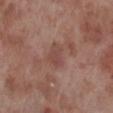Recorded during total-body skin imaging; not selected for excision or biopsy. A male patient, aged approximately 70. Automated tile analysis of the lesion measured a shape eccentricity near 0.7 and two-axis asymmetry of about 0.3. It also reported a border-irregularity rating of about 2.5/10 and a peripheral color-asymmetry measure near 1. The software also gave an automated nevus-likeness rating near 0 out of 100 and lesion-presence confidence of about 100/100. This is a white-light tile. The recorded lesion diameter is about 3 mm. A lesion tile, about 15 mm wide, cut from a 3D total-body photograph. The lesion is on the right lower leg.